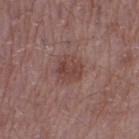Q: Was this lesion biopsied?
A: catalogued during a skin exam; not biopsied
Q: Automated lesion metrics?
A: a lesion area of about 7 mm², a shape eccentricity near 0.45, and a symmetry-axis asymmetry near 0.15; a border-irregularity rating of about 2/10, internal color variation of about 3 on a 0–10 scale, and a peripheral color-asymmetry measure near 1
Q: What is the lesion's diameter?
A: ~3.5 mm (longest diameter)
Q: What is the imaging modality?
A: ~15 mm crop, total-body skin-cancer survey
Q: What are the patient's age and sex?
A: male, aged around 50
Q: What is the anatomic site?
A: the left thigh